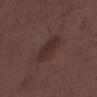  biopsy_status: not biopsied; imaged during a skin examination
  lesion_size:
    long_diameter_mm_approx: 4.5
  image:
    source: total-body photography crop
    field_of_view_mm: 15
  lighting: white-light
  patient:
    sex: male
    age_approx: 50